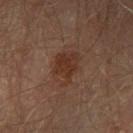Imaged during a routine full-body skin examination; the lesion was not biopsied and no histopathology is available.
A male subject, in their mid- to late 60s.
Longest diameter approximately 4.5 mm.
A 15 mm close-up extracted from a 3D total-body photography capture.
The lesion is located on the left thigh.